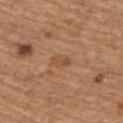Case summary:
* biopsy status: imaged on a skin check; not biopsied
* subject: male, aged around 70
* site: the upper back
* automated metrics: a border-irregularity rating of about 3/10, internal color variation of about 1.5 on a 0–10 scale, and peripheral color asymmetry of about 0.5
* lesion diameter: about 2.5 mm
* illumination: white-light illumination
* image source: ~15 mm tile from a whole-body skin photo